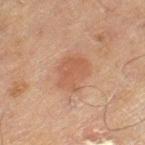Notes:
• biopsy status · catalogued during a skin exam; not biopsied
• subject · male, aged 68 to 72
• anatomic site · the leg
• imaging modality · ~15 mm crop, total-body skin-cancer survey